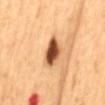Captured during whole-body skin photography for melanoma surveillance; the lesion was not biopsied.
A close-up tile cropped from a whole-body skin photograph, about 15 mm across.
This is a cross-polarized tile.
A male subject aged approximately 65.
From the mid back.